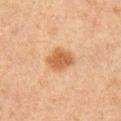The lesion was tiled from a total-body skin photograph and was not biopsied. The lesion is on the right upper arm. The tile uses cross-polarized illumination. The recorded lesion diameter is about 4 mm. The patient is a male aged around 50. A region of skin cropped from a whole-body photographic capture, roughly 15 mm wide.